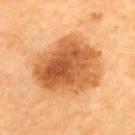biopsy status: catalogued during a skin exam; not biopsied
lesion diameter: ~8.5 mm (longest diameter)
lighting: cross-polarized illumination
image-analysis metrics: an automated nevus-likeness rating near 80 out of 100
image source: ~15 mm tile from a whole-body skin photo
anatomic site: the back
patient: aged 68–72